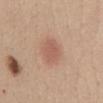No biopsy was performed on this lesion — it was imaged during a full skin examination and was not determined to be concerning.
The patient is a female in their 50s.
A close-up tile cropped from a whole-body skin photograph, about 15 mm across.
On the abdomen.
Automated image analysis of the tile measured an area of roughly 6.5 mm², an eccentricity of roughly 0.7, and a shape-asymmetry score of about 0.2 (0 = symmetric). And it measured a lesion color around L≈57 a*≈22 b*≈29 in CIELAB, about 8 CIELAB-L* units darker than the surrounding skin, and a normalized lesion–skin contrast near 5.5. The software also gave a border-irregularity index near 1.5/10, internal color variation of about 1.5 on a 0–10 scale, and radial color variation of about 0.5.
Longest diameter approximately 3.5 mm.